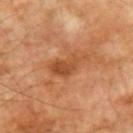Clinical summary: Longest diameter approximately 4.5 mm. The tile uses cross-polarized illumination. A close-up tile cropped from a whole-body skin photograph, about 15 mm across. A male subject, aged approximately 70. The lesion is located on the left upper arm.